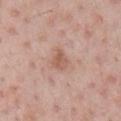| key | value |
|---|---|
| follow-up | imaged on a skin check; not biopsied |
| image | total-body-photography crop, ~15 mm field of view |
| patient | male, aged around 50 |
| lesion size | about 2.5 mm |
| illumination | white-light illumination |
| image-analysis metrics | a shape-asymmetry score of about 0.35 (0 = symmetric); a color-variation rating of about 3/10 and peripheral color asymmetry of about 1; a nevus-likeness score of about 0/100 and a detector confidence of about 100 out of 100 that the crop contains a lesion |
| location | the chest |